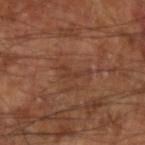image:
  source: total-body photography crop
  field_of_view_mm: 15
lesion_size:
  long_diameter_mm_approx: 3.0
patient:
  sex: male
  age_approx: 65
automated_metrics:
  area_mm2_approx: 3.0
  eccentricity: 0.9
  shape_asymmetry: 0.7
  vs_skin_darker_L: 6.0
  border_irregularity_0_10: 8.5
  peripheral_color_asymmetry: 0.0
lighting: cross-polarized
site: left arm